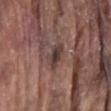Captured during whole-body skin photography for melanoma surveillance; the lesion was not biopsied. A male patient in their 80s. The tile uses white-light illumination. A region of skin cropped from a whole-body photographic capture, roughly 15 mm wide. Automated tile analysis of the lesion measured an area of roughly 5.5 mm², an outline eccentricity of about 0.8 (0 = round, 1 = elongated), and a shape-asymmetry score of about 0.25 (0 = symmetric). It also reported a border-irregularity index near 2.5/10, a color-variation rating of about 3.5/10, and radial color variation of about 1. Located on the abdomen.